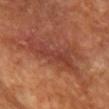Impression: Recorded during total-body skin imaging; not selected for excision or biopsy. Image and clinical context: A region of skin cropped from a whole-body photographic capture, roughly 15 mm wide. A female patient, aged approximately 75. Captured under cross-polarized illumination. The lesion is on the head or neck. About 8.5 mm across. The lesion-visualizer software estimated an area of roughly 17 mm² and a shape eccentricity near 0.95. The analysis additionally found a within-lesion color-variation index near 4/10. The software also gave a detector confidence of about 75 out of 100 that the crop contains a lesion.